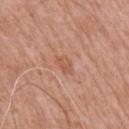Assessment:
Part of a total-body skin-imaging series; this lesion was reviewed on a skin check and was not flagged for biopsy.
Clinical summary:
This is a white-light tile. A male subject in their 60s. From the left upper arm. A 15 mm crop from a total-body photograph taken for skin-cancer surveillance. Longest diameter approximately 2.5 mm.